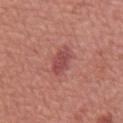follow-up = total-body-photography surveillance lesion; no biopsy
size = about 3.5 mm
lighting = white-light illumination
site = the mid back
TBP lesion metrics = an area of roughly 6.5 mm²; an average lesion color of about L≈49 a*≈29 b*≈24 (CIELAB) and a lesion–skin lightness drop of about 9; an automated nevus-likeness rating near 40 out of 100 and a detector confidence of about 100 out of 100 that the crop contains a lesion
subject = male, aged 63–67
acquisition = ~15 mm tile from a whole-body skin photo